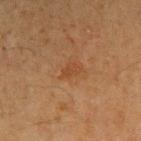Case summary:
– biopsy status · no biopsy performed (imaged during a skin exam)
– lesion size · about 2.5 mm
– illumination · cross-polarized
– image · ~15 mm tile from a whole-body skin photo
– automated lesion analysis · an average lesion color of about L≈40 a*≈21 b*≈33 (CIELAB) and a lesion–skin lightness drop of about 5
– subject · male, roughly 55 years of age
– body site · the left arm